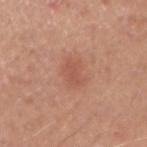biopsy status — imaged on a skin check; not biopsied
site — the right upper arm
image source — 15 mm crop, total-body photography
lesion size — ≈3 mm
TBP lesion metrics — an area of roughly 3.5 mm², a shape eccentricity near 0.85, and two-axis asymmetry of about 0.3; a border-irregularity index near 3.5/10, a within-lesion color-variation index near 1/10, and a peripheral color-asymmetry measure near 0.5; an automated nevus-likeness rating near 65 out of 100 and a lesion-detection confidence of about 100/100
subject — male, aged around 40
illumination — white-light illumination